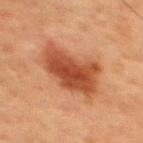Captured during whole-body skin photography for melanoma surveillance; the lesion was not biopsied. From the upper back. A male subject, aged 58 to 62. A lesion tile, about 15 mm wide, cut from a 3D total-body photograph.